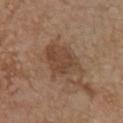Impression: Captured during whole-body skin photography for melanoma surveillance; the lesion was not biopsied. Image and clinical context: The tile uses white-light illumination. On the chest. Approximately 5 mm at its widest. A 15 mm crop from a total-body photograph taken for skin-cancer surveillance. A female patient in their mid-60s. Automated tile analysis of the lesion measured a shape eccentricity near 0.65 and a symmetry-axis asymmetry near 0.25.